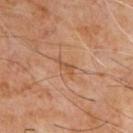No biopsy was performed on this lesion — it was imaged during a full skin examination and was not determined to be concerning.